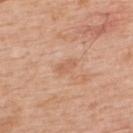Automated image analysis of the tile measured a within-lesion color-variation index near 0.5/10. The software also gave an automated nevus-likeness rating near 0 out of 100 and a detector confidence of about 100 out of 100 that the crop contains a lesion. This image is a 15 mm lesion crop taken from a total-body photograph. The lesion is on the upper back. A male patient aged 58–62.